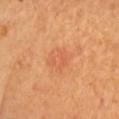| key | value |
|---|---|
| subject | female, aged around 65 |
| imaging modality | ~15 mm crop, total-body skin-cancer survey |
| diameter | ~3 mm (longest diameter) |
| body site | the head or neck |
| automated metrics | a shape-asymmetry score of about 0.2 (0 = symmetric) |
| tile lighting | cross-polarized illumination |
| histopathology | an actinic keratosis — a borderline lesion of uncertain malignant potential |